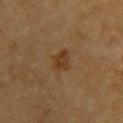<case>
  <biopsy_status>not biopsied; imaged during a skin examination</biopsy_status>
  <lesion_size>
    <long_diameter_mm_approx>2.5</long_diameter_mm_approx>
  </lesion_size>
  <automated_metrics>
    <vs_skin_contrast_norm>7.5</vs_skin_contrast_norm>
  </automated_metrics>
  <site>upper back</site>
  <image>
    <source>total-body photography crop</source>
    <field_of_view_mm>15</field_of_view_mm>
  </image>
  <patient>
    <sex>male</sex>
    <age_approx>85+</age_approx>
  </patient>
  <lighting>cross-polarized</lighting>
</case>